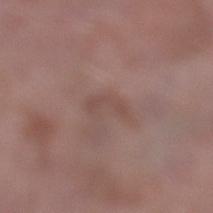The lesion was tiled from a total-body skin photograph and was not biopsied. The recorded lesion diameter is about 3.5 mm. A 15 mm crop from a total-body photograph taken for skin-cancer surveillance. A female subject about 70 years old. Imaged with white-light lighting. On the left lower leg.